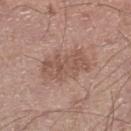Imaged during a routine full-body skin examination; the lesion was not biopsied and no histopathology is available.
Automated image analysis of the tile measured a border-irregularity index near 4/10, a color-variation rating of about 3/10, and a peripheral color-asymmetry measure near 1. It also reported a detector confidence of about 100 out of 100 that the crop contains a lesion.
The lesion is on the left lower leg.
A 15 mm crop from a total-body photograph taken for skin-cancer surveillance.
Imaged with white-light lighting.
A male subject aged approximately 55.